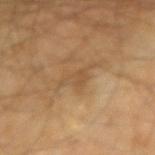No biopsy was performed on this lesion — it was imaged during a full skin examination and was not determined to be concerning. This image is a 15 mm lesion crop taken from a total-body photograph. A male patient about 60 years old. From the right upper arm.